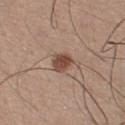  biopsy_status: not biopsied; imaged during a skin examination
  patient:
    sex: male
    age_approx: 25
  lesion_size:
    long_diameter_mm_approx: 2.5
  image:
    source: total-body photography crop
    field_of_view_mm: 15
  automated_metrics:
    area_mm2_approx: 5.0
    shape_asymmetry: 0.25
    cielab_L: 47
    cielab_a: 19
    cielab_b: 27
    vs_skin_darker_L: 13.0
    vs_skin_contrast_norm: 9.5
  site: chest
  lighting: white-light